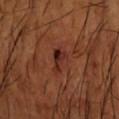| key | value |
|---|---|
| workup | imaged on a skin check; not biopsied |
| lighting | cross-polarized |
| body site | the right forearm |
| imaging modality | total-body-photography crop, ~15 mm field of view |
| patient | male, aged 63 to 67 |